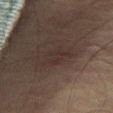Recorded during total-body skin imaging; not selected for excision or biopsy. This image is a 15 mm lesion crop taken from a total-body photograph. A male patient aged approximately 75. This is a cross-polarized tile. The lesion is located on the right thigh. About 3.5 mm across.